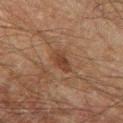{
  "lesion_size": {
    "long_diameter_mm_approx": 3.5
  },
  "lighting": "cross-polarized",
  "site": "leg",
  "image": {
    "source": "total-body photography crop",
    "field_of_view_mm": 15
  },
  "patient": {
    "sex": "male",
    "age_approx": 60
  }
}